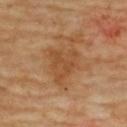notes — imaged on a skin check; not biopsied | image — 15 mm crop, total-body photography | location — the upper back | lesion diameter — about 4.5 mm | patient — female, aged approximately 60.